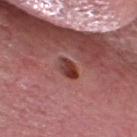Acquisition and patient details: A male subject, approximately 70 years of age. On the head or neck. The recorded lesion diameter is about 3 mm. Cropped from a whole-body photographic skin survey; the tile spans about 15 mm.